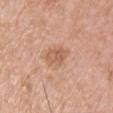Captured during whole-body skin photography for melanoma surveillance; the lesion was not biopsied. The lesion's longest dimension is about 3.5 mm. From the front of the torso. The tile uses white-light illumination. A male subject approximately 65 years of age. A lesion tile, about 15 mm wide, cut from a 3D total-body photograph.